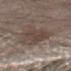Q: Lesion size?
A: ~5.5 mm (longest diameter)
Q: What is the anatomic site?
A: the left forearm
Q: What kind of image is this?
A: 15 mm crop, total-body photography
Q: What are the patient's age and sex?
A: male, in their mid-50s
Q: How was the tile lit?
A: white-light